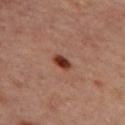No biopsy was performed on this lesion — it was imaged during a full skin examination and was not determined to be concerning. A 15 mm close-up extracted from a 3D total-body photography capture. A female patient approximately 45 years of age. Located on the upper back. The tile uses cross-polarized illumination.